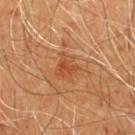site: the chest | lesion size: ~3 mm (longest diameter) | lighting: cross-polarized illumination | imaging modality: 15 mm crop, total-body photography | subject: male, aged approximately 60.